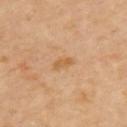notes: catalogued during a skin exam; not biopsied
illumination: cross-polarized
diameter: ≈2.5 mm
subject: female, aged 63–67
location: the left upper arm
imaging modality: total-body-photography crop, ~15 mm field of view